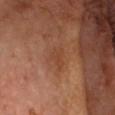notes = imaged on a skin check; not biopsied | lesion diameter = ~2.5 mm (longest diameter) | patient = male, aged 53–57 | image-analysis metrics = a lesion area of about 3.5 mm², an eccentricity of roughly 0.75, and a symmetry-axis asymmetry near 0.4; a lesion color around L≈42 a*≈23 b*≈32 in CIELAB, about 5 CIELAB-L* units darker than the surrounding skin, and a normalized lesion–skin contrast near 5; a detector confidence of about 100 out of 100 that the crop contains a lesion | imaging modality = ~15 mm tile from a whole-body skin photo | location = the head or neck | tile lighting = cross-polarized.